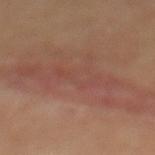The subject is a male approximately 60 years of age. The lesion is located on the abdomen. The lesion's longest dimension is about 14 mm. This is a cross-polarized tile. A region of skin cropped from a whole-body photographic capture, roughly 15 mm wide.